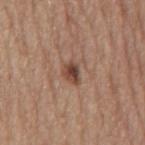Recorded during total-body skin imaging; not selected for excision or biopsy.